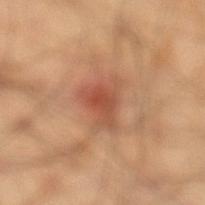Notes:
– subject — male, roughly 40 years of age
– image — 15 mm crop, total-body photography
– body site — the left lower leg
– tile lighting — cross-polarized
– size — ≈4 mm